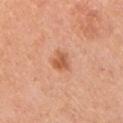{
  "biopsy_status": "not biopsied; imaged during a skin examination",
  "site": "chest",
  "automated_metrics": {
    "eccentricity": 0.5,
    "shape_asymmetry": 0.3,
    "cielab_L": 59,
    "cielab_a": 27,
    "cielab_b": 37,
    "vs_skin_contrast_norm": 7.5,
    "border_irregularity_0_10": 3.0,
    "color_variation_0_10": 2.5,
    "peripheral_color_asymmetry": 1.0
  },
  "lesion_size": {
    "long_diameter_mm_approx": 2.5
  },
  "image": {
    "source": "total-body photography crop",
    "field_of_view_mm": 15
  },
  "lighting": "white-light",
  "patient": {
    "sex": "male",
    "age_approx": 50
  }
}